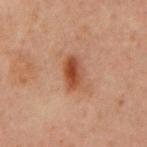Clinical impression: This lesion was catalogued during total-body skin photography and was not selected for biopsy. Clinical summary: The tile uses cross-polarized illumination. A male subject, aged approximately 60. Located on the front of the torso. A close-up tile cropped from a whole-body skin photograph, about 15 mm across.